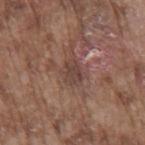Captured during whole-body skin photography for melanoma surveillance; the lesion was not biopsied. The recorded lesion diameter is about 3.5 mm. The lesion is located on the mid back. The tile uses white-light illumination. A region of skin cropped from a whole-body photographic capture, roughly 15 mm wide. A male patient, in their mid-70s.